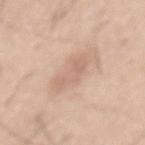No biopsy was performed on this lesion — it was imaged during a full skin examination and was not determined to be concerning.
A male patient in their mid- to late 40s.
The lesion-visualizer software estimated a border-irregularity rating of about 5/10, a within-lesion color-variation index near 2.5/10, and peripheral color asymmetry of about 1.
This is a white-light tile.
The lesion is located on the mid back.
A 15 mm close-up tile from a total-body photography series done for melanoma screening.